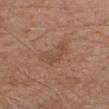workup: imaged on a skin check; not biopsied
lesion size: ≈4 mm
automated lesion analysis: a lesion color around L≈49 a*≈20 b*≈29 in CIELAB, about 6 CIELAB-L* units darker than the surrounding skin, and a lesion-to-skin contrast of about 4.5 (normalized; higher = more distinct)
illumination: white-light illumination
body site: the right upper arm
patient: male, aged around 65
image: ~15 mm crop, total-body skin-cancer survey